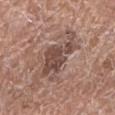This lesion was catalogued during total-body skin photography and was not selected for biopsy. Cropped from a total-body skin-imaging series; the visible field is about 15 mm. Located on the left lower leg. Imaged with white-light lighting. Automated image analysis of the tile measured a border-irregularity rating of about 8/10, a within-lesion color-variation index near 2.5/10, and peripheral color asymmetry of about 1. And it measured an automated nevus-likeness rating near 25 out of 100 and a lesion-detection confidence of about 100/100. A female patient aged approximately 75.